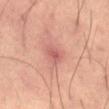Findings:
– follow-up: catalogued during a skin exam; not biopsied
– body site: the left thigh
– size: ~2.5 mm (longest diameter)
– acquisition: total-body-photography crop, ~15 mm field of view
– lighting: cross-polarized
– automated lesion analysis: an area of roughly 4.5 mm², an outline eccentricity of about 0.6 (0 = round, 1 = elongated), and a shape-asymmetry score of about 0.3 (0 = symmetric); a normalized lesion–skin contrast near 6
– patient: male, aged 58–62